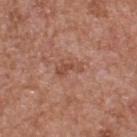follow-up: catalogued during a skin exam; not biopsied | site: the upper back | lesion diameter: about 3 mm | imaging modality: 15 mm crop, total-body photography | subject: male, in their mid- to late 60s | illumination: white-light illumination.